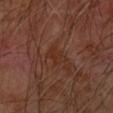This lesion was catalogued during total-body skin photography and was not selected for biopsy. Longest diameter approximately 2.5 mm. The lesion is located on the arm. Cropped from a total-body skin-imaging series; the visible field is about 15 mm. Imaged with cross-polarized lighting. The subject is a male aged 68–72.